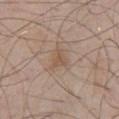Impression: No biopsy was performed on this lesion — it was imaged during a full skin examination and was not determined to be concerning. Image and clinical context: The lesion is located on the chest. The recorded lesion diameter is about 3 mm. The patient is a male approximately 55 years of age. A roughly 15 mm field-of-view crop from a total-body skin photograph.